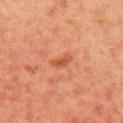{"lighting": "cross-polarized", "lesion_size": {"long_diameter_mm_approx": 3.0}, "image": {"source": "total-body photography crop", "field_of_view_mm": 15}, "site": "right upper arm", "patient": {"sex": "female", "age_approx": 55}}